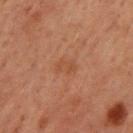biopsy status = imaged on a skin check; not biopsied.